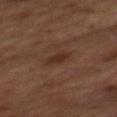The lesion was photographed on a routine skin check and not biopsied; there is no pathology result. A lesion tile, about 15 mm wide, cut from a 3D total-body photograph. The lesion is located on the back. Imaged with cross-polarized lighting. A male subject aged approximately 65. Longest diameter approximately 3 mm. The lesion-visualizer software estimated a lesion area of about 4.5 mm² and a shape-asymmetry score of about 0.2 (0 = symmetric). And it measured a border-irregularity rating of about 2/10, a color-variation rating of about 2/10, and a peripheral color-asymmetry measure near 0.5. It also reported an automated nevus-likeness rating near 85 out of 100 and lesion-presence confidence of about 100/100.